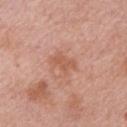No biopsy was performed on this lesion — it was imaged during a full skin examination and was not determined to be concerning. Automated tile analysis of the lesion measured border irregularity of about 3.5 on a 0–10 scale, a within-lesion color-variation index near 1/10, and peripheral color asymmetry of about 0.5. A male patient, aged 53 to 57. The lesion's longest dimension is about 3 mm. A 15 mm crop from a total-body photograph taken for skin-cancer surveillance. From the arm.